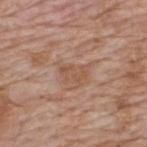| field | value |
|---|---|
| anatomic site | the upper back |
| patient | male, aged 58–62 |
| automated metrics | an area of roughly 5 mm², an outline eccentricity of about 0.8 (0 = round, 1 = elongated), and a shape-asymmetry score of about 0.3 (0 = symmetric) |
| lesion diameter | about 3 mm |
| lighting | white-light illumination |
| acquisition | total-body-photography crop, ~15 mm field of view |